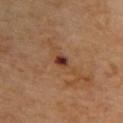Recorded during total-body skin imaging; not selected for excision or biopsy.
Approximately 2 mm at its widest.
A close-up tile cropped from a whole-body skin photograph, about 15 mm across.
The patient is a female aged around 70.
Located on the upper back.
Automated tile analysis of the lesion measured a mean CIELAB color near L≈34 a*≈24 b*≈25, about 14 CIELAB-L* units darker than the surrounding skin, and a normalized border contrast of about 13. It also reported a border-irregularity rating of about 2/10. And it measured a nevus-likeness score of about 10/100 and a lesion-detection confidence of about 100/100.
This is a cross-polarized tile.